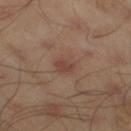Acquisition and patient details:
The patient is a male in their mid- to late 40s. This is a cross-polarized tile. From the right thigh. A close-up tile cropped from a whole-body skin photograph, about 15 mm across. The lesion-visualizer software estimated an average lesion color of about L≈43 a*≈18 b*≈24 (CIELAB), a lesion–skin lightness drop of about 6, and a normalized lesion–skin contrast near 4.5.